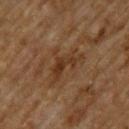The lesion was tiled from a total-body skin photograph and was not biopsied. A roughly 15 mm field-of-view crop from a total-body skin photograph. The patient is a male in their mid-60s. The total-body-photography lesion software estimated a mean CIELAB color near L≈31 a*≈17 b*≈29 and a lesion-to-skin contrast of about 7 (normalized; higher = more distinct). And it measured border irregularity of about 9 on a 0–10 scale, a within-lesion color-variation index near 3.5/10, and a peripheral color-asymmetry measure near 1. On the upper back. Measured at roughly 5 mm in maximum diameter.